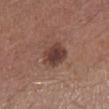| field | value |
|---|---|
| workup | catalogued during a skin exam; not biopsied |
| body site | the left lower leg |
| subject | male, in their mid-60s |
| acquisition | 15 mm crop, total-body photography |